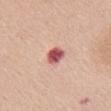This lesion was catalogued during total-body skin photography and was not selected for biopsy. This is a white-light tile. The lesion is on the chest. A female subject approximately 65 years of age. Cropped from a whole-body photographic skin survey; the tile spans about 15 mm. Measured at roughly 2.5 mm in maximum diameter. Automated tile analysis of the lesion measured a mean CIELAB color near L≈55 a*≈31 b*≈23, a lesion–skin lightness drop of about 18, and a normalized lesion–skin contrast near 11.5.